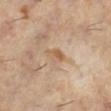{
  "biopsy_status": "not biopsied; imaged during a skin examination",
  "patient": {
    "sex": "female",
    "age_approx": 60
  },
  "site": "left lower leg",
  "lesion_size": {
    "long_diameter_mm_approx": 2.5
  },
  "lighting": "cross-polarized",
  "image": {
    "source": "total-body photography crop",
    "field_of_view_mm": 15
  }
}